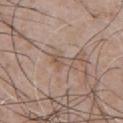This lesion was catalogued during total-body skin photography and was not selected for biopsy. The lesion is on the chest. The tile uses white-light illumination. The subject is a male about 50 years old. Automated tile analysis of the lesion measured an outline eccentricity of about 0.85 (0 = round, 1 = elongated) and two-axis asymmetry of about 0.45. The software also gave a mean CIELAB color near L≈54 a*≈15 b*≈26, a lesion–skin lightness drop of about 6, and a lesion-to-skin contrast of about 5 (normalized; higher = more distinct). A region of skin cropped from a whole-body photographic capture, roughly 15 mm wide.